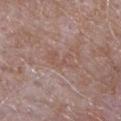follow-up: imaged on a skin check; not biopsied
patient: male, roughly 65 years of age
anatomic site: the chest
automated lesion analysis: a mean CIELAB color near L≈53 a*≈18 b*≈24, about 6 CIELAB-L* units darker than the surrounding skin, and a normalized border contrast of about 4.5; an automated nevus-likeness rating near 0 out of 100
diameter: about 4.5 mm
imaging modality: 15 mm crop, total-body photography
illumination: white-light illumination